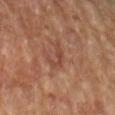Captured during whole-body skin photography for melanoma surveillance; the lesion was not biopsied. Automated tile analysis of the lesion measured a mean CIELAB color near L≈43 a*≈23 b*≈28 and a normalized lesion–skin contrast near 5.5. The software also gave border irregularity of about 7.5 on a 0–10 scale, a color-variation rating of about 0/10, and radial color variation of about 0. This is a cross-polarized tile. A lesion tile, about 15 mm wide, cut from a 3D total-body photograph. A female subject, aged 68–72. The lesion is on the left forearm.